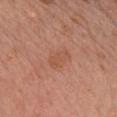workup: catalogued during a skin exam; not biopsied | imaging modality: ~15 mm crop, total-body skin-cancer survey | image-analysis metrics: a lesion area of about 5.5 mm², an outline eccentricity of about 0.75 (0 = round, 1 = elongated), and a shape-asymmetry score of about 0.3 (0 = symmetric); a lesion color around L≈54 a*≈25 b*≈32 in CIELAB, a lesion–skin lightness drop of about 5, and a lesion-to-skin contrast of about 4.5 (normalized; higher = more distinct); a peripheral color-asymmetry measure near 0.5; a nevus-likeness score of about 0/100 and a lesion-detection confidence of about 100/100 | subject: female, about 60 years old | body site: the chest | illumination: white-light illumination.